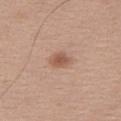Impression: Part of a total-body skin-imaging series; this lesion was reviewed on a skin check and was not flagged for biopsy. Clinical summary: On the abdomen. Captured under white-light illumination. A male patient in their mid-70s. Longest diameter approximately 2.5 mm. A lesion tile, about 15 mm wide, cut from a 3D total-body photograph.